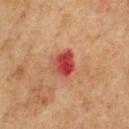The lesion was photographed on a routine skin check and not biopsied; there is no pathology result.
The recorded lesion diameter is about 3 mm.
Automated image analysis of the tile measured a lesion color around L≈36 a*≈32 b*≈26 in CIELAB and a lesion–skin lightness drop of about 12.
The tile uses cross-polarized illumination.
Located on the chest.
A 15 mm close-up tile from a total-body photography series done for melanoma screening.
A male patient, about 75 years old.